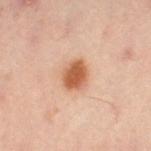Recorded during total-body skin imaging; not selected for excision or biopsy.